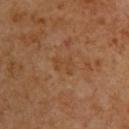notes — imaged on a skin check; not biopsied
image source — total-body-photography crop, ~15 mm field of view
lighting — cross-polarized
image-analysis metrics — an area of roughly 4.5 mm², an outline eccentricity of about 0.65 (0 = round, 1 = elongated), and a shape-asymmetry score of about 0.3 (0 = symmetric); roughly 5 lightness units darker than nearby skin; border irregularity of about 3.5 on a 0–10 scale and a color-variation rating of about 2/10; a lesion-detection confidence of about 100/100
subject — male, aged approximately 60
lesion size — ~3 mm (longest diameter)
location — the upper back